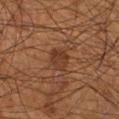Clinical impression:
Recorded during total-body skin imaging; not selected for excision or biopsy.
Image and clinical context:
Located on the leg. Captured under cross-polarized illumination. This image is a 15 mm lesion crop taken from a total-body photograph. The patient is a male approximately 55 years of age. About 3 mm across.